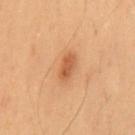Case summary:
- biopsy status: imaged on a skin check; not biopsied
- imaging modality: ~15 mm tile from a whole-body skin photo
- lesion size: ~3 mm (longest diameter)
- subject: male, aged approximately 55
- tile lighting: cross-polarized illumination
- anatomic site: the mid back